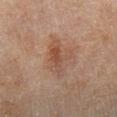Impression:
Captured during whole-body skin photography for melanoma surveillance; the lesion was not biopsied.
Context:
A female subject aged around 60. Automated tile analysis of the lesion measured an area of roughly 8.5 mm² and a shape-asymmetry score of about 0.4 (0 = symmetric). And it measured an automated nevus-likeness rating near 30 out of 100. Approximately 4.5 mm at its widest. A 15 mm crop from a total-body photograph taken for skin-cancer surveillance. Located on the right lower leg.